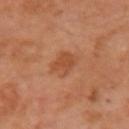patient:
  sex: female
  age_approx: 55
lesion_size:
  long_diameter_mm_approx: 2.5
image:
  source: total-body photography crop
  field_of_view_mm: 15
site: arm
lighting: cross-polarized